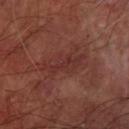Case summary:
- tile lighting — cross-polarized
- TBP lesion metrics — a lesion area of about 9 mm², a shape eccentricity near 0.95, and a symmetry-axis asymmetry near 0.35
- patient — male, aged around 65
- image — 15 mm crop, total-body photography
- size — ≈5.5 mm
- location — the left forearm